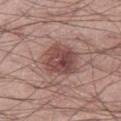<record>
<biopsy_status>not biopsied; imaged during a skin examination</biopsy_status>
<lesion_size>
  <long_diameter_mm_approx>4.5</long_diameter_mm_approx>
</lesion_size>
<site>right thigh</site>
<lighting>white-light</lighting>
<image>
  <source>total-body photography crop</source>
  <field_of_view_mm>15</field_of_view_mm>
</image>
<patient>
  <sex>male</sex>
  <age_approx>20</age_approx>
</patient>
</record>